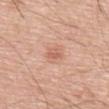Clinical impression:
This lesion was catalogued during total-body skin photography and was not selected for biopsy.